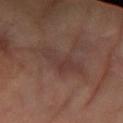Imaged during a routine full-body skin examination; the lesion was not biopsied and no histopathology is available.
About 5 mm across.
The patient is a female in their 70s.
A 15 mm close-up tile from a total-body photography series done for melanoma screening.
From the left forearm.
The tile uses cross-polarized illumination.
Automated tile analysis of the lesion measured an area of roughly 8.5 mm², a shape eccentricity near 0.9, and two-axis asymmetry of about 0.4. And it measured a mean CIELAB color near L≈37 a*≈19 b*≈22, roughly 6 lightness units darker than nearby skin, and a normalized border contrast of about 5. It also reported an automated nevus-likeness rating near 0 out of 100 and a lesion-detection confidence of about 95/100.